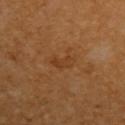follow-up: catalogued during a skin exam; not biopsied | tile lighting: cross-polarized illumination | automated metrics: a lesion area of about 4 mm² and a shape-asymmetry score of about 0.3 (0 = symmetric); a lesion color around L≈36 a*≈21 b*≈34 in CIELAB, roughly 5 lightness units darker than nearby skin, and a normalized border contrast of about 5; a border-irregularity rating of about 3/10 | location: the left upper arm | patient: male, aged around 60 | image: ~15 mm crop, total-body skin-cancer survey | lesion size: about 2.5 mm.